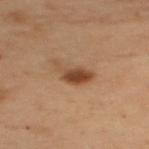Part of a total-body skin-imaging series; this lesion was reviewed on a skin check and was not flagged for biopsy. The total-body-photography lesion software estimated an area of roughly 6.5 mm², an eccentricity of roughly 0.85, and a shape-asymmetry score of about 0.5 (0 = symmetric). The analysis additionally found a lesion color around L≈38 a*≈18 b*≈28 in CIELAB. The software also gave a lesion-detection confidence of about 100/100. The lesion is on the upper back. This image is a 15 mm lesion crop taken from a total-body photograph. Longest diameter approximately 4 mm. A female patient aged approximately 55.